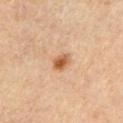Case summary:
• notes — imaged on a skin check; not biopsied
• location — the left upper arm
• tile lighting — cross-polarized illumination
• image — ~15 mm tile from a whole-body skin photo
• patient — male, about 55 years old
• size — ≈2.5 mm
• automated lesion analysis — a color-variation rating of about 5/10; a detector confidence of about 100 out of 100 that the crop contains a lesion